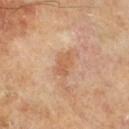{"site": "right lower leg", "patient": {"sex": "male", "age_approx": 65}, "image": {"source": "total-body photography crop", "field_of_view_mm": 15}}